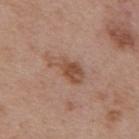Clinical impression: Imaged during a routine full-body skin examination; the lesion was not biopsied and no histopathology is available. Image and clinical context: A male subject in their mid- to late 50s. Located on the mid back. This image is a 15 mm lesion crop taken from a total-body photograph.